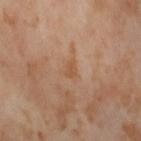Findings:
- workup: imaged on a skin check; not biopsied
- imaging modality: total-body-photography crop, ~15 mm field of view
- body site: the right thigh
- patient: female, aged 53–57
- lighting: cross-polarized illumination